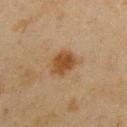Q: Was a biopsy performed?
A: imaged on a skin check; not biopsied
Q: How large is the lesion?
A: ≈3.5 mm
Q: Who is the patient?
A: male, roughly 45 years of age
Q: What is the imaging modality?
A: 15 mm crop, total-body photography
Q: What is the anatomic site?
A: the left upper arm
Q: Automated lesion metrics?
A: a footprint of about 8 mm², a shape eccentricity near 0.65, and two-axis asymmetry of about 0.15; a mean CIELAB color near L≈39 a*≈16 b*≈31, about 9 CIELAB-L* units darker than the surrounding skin, and a normalized border contrast of about 9; radial color variation of about 1; an automated nevus-likeness rating near 95 out of 100